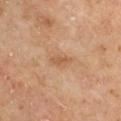A 15 mm close-up tile from a total-body photography series done for melanoma screening. The tile uses cross-polarized illumination. The lesion's longest dimension is about 2.5 mm. An algorithmic analysis of the crop reported an eccentricity of roughly 0.85. The software also gave a border-irregularity index near 2.5/10, a within-lesion color-variation index near 1.5/10, and a peripheral color-asymmetry measure near 0.5. The analysis additionally found an automated nevus-likeness rating near 0 out of 100 and a detector confidence of about 100 out of 100 that the crop contains a lesion. The patient is a male in their mid-60s. The lesion is on the left upper arm.